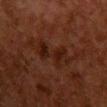image: 15 mm crop, total-body photography
automated lesion analysis: a lesion color around L≈17 a*≈18 b*≈21 in CIELAB, about 5 CIELAB-L* units darker than the surrounding skin, and a lesion-to-skin contrast of about 6 (normalized; higher = more distinct); a border-irregularity index near 8.5/10, a color-variation rating of about 4/10, and a peripheral color-asymmetry measure near 1
subject: female, in their 50s
location: the chest
illumination: cross-polarized